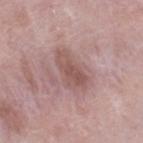Case summary:
– follow-up: total-body-photography surveillance lesion; no biopsy
– site: the leg
– illumination: white-light illumination
– automated lesion analysis: a mean CIELAB color near L≈53 a*≈20 b*≈21 and a normalized border contrast of about 6.5; a border-irregularity rating of about 4/10, internal color variation of about 3.5 on a 0–10 scale, and peripheral color asymmetry of about 1.5
– diameter: ≈5 mm
– acquisition: total-body-photography crop, ~15 mm field of view
– patient: male, about 40 years old